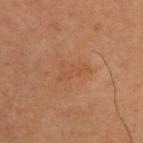Imaged during a routine full-body skin examination; the lesion was not biopsied and no histopathology is available. Automated image analysis of the tile measured a footprint of about 5.5 mm², an outline eccentricity of about 0.85 (0 = round, 1 = elongated), and a shape-asymmetry score of about 0.6 (0 = symmetric). The analysis additionally found a lesion color around L≈49 a*≈23 b*≈34 in CIELAB, about 4 CIELAB-L* units darker than the surrounding skin, and a normalized border contrast of about 4. It also reported a border-irregularity index near 6.5/10 and internal color variation of about 1 on a 0–10 scale. A male subject in their mid-60s. The lesion is on the upper back. The recorded lesion diameter is about 3.5 mm. This is a cross-polarized tile. A 15 mm close-up tile from a total-body photography series done for melanoma screening.